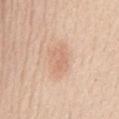• follow-up: imaged on a skin check; not biopsied
• image-analysis metrics: a lesion area of about 7.5 mm², an outline eccentricity of about 0.7 (0 = round, 1 = elongated), and two-axis asymmetry of about 0.3; a color-variation rating of about 2.5/10 and radial color variation of about 1; a nevus-likeness score of about 20/100 and a lesion-detection confidence of about 100/100
• patient: female, aged approximately 75
• image: ~15 mm tile from a whole-body skin photo
• lighting: white-light illumination
• diameter: about 3.5 mm
• site: the mid back